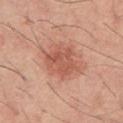Assessment: The lesion was tiled from a total-body skin photograph and was not biopsied. Context: A roughly 15 mm field-of-view crop from a total-body skin photograph. An algorithmic analysis of the crop reported an outline eccentricity of about 0.5 (0 = round, 1 = elongated) and a shape-asymmetry score of about 0.25 (0 = symmetric). The software also gave border irregularity of about 3 on a 0–10 scale, a within-lesion color-variation index near 3.5/10, and radial color variation of about 1.5. Located on the chest. A male patient aged around 45.